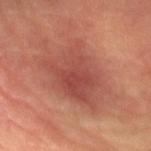Captured during whole-body skin photography for melanoma surveillance; the lesion was not biopsied. Approximately 7 mm at its widest. On the left arm. Automated image analysis of the tile measured lesion-presence confidence of about 95/100. A male patient, roughly 65 years of age. A 15 mm crop from a total-body photograph taken for skin-cancer surveillance.